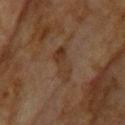This lesion was catalogued during total-body skin photography and was not selected for biopsy.
The lesion is on the head or neck.
A female patient, aged 58 to 62.
This image is a 15 mm lesion crop taken from a total-body photograph.